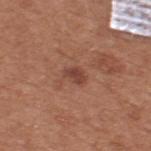Assessment: The lesion was photographed on a routine skin check and not biopsied; there is no pathology result. Clinical summary: A male patient in their mid-50s. The lesion is on the upper back. The tile uses white-light illumination. This image is a 15 mm lesion crop taken from a total-body photograph. The lesion's longest dimension is about 2.5 mm. The lesion-visualizer software estimated a footprint of about 3 mm². The analysis additionally found a mean CIELAB color near L≈43 a*≈24 b*≈27 and a lesion–skin lightness drop of about 9. The software also gave a border-irregularity index near 3/10 and peripheral color asymmetry of about 0.5.